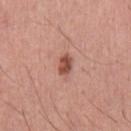Q: Was this lesion biopsied?
A: no biopsy performed (imaged during a skin exam)